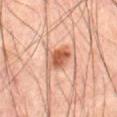Imaged during a routine full-body skin examination; the lesion was not biopsied and no histopathology is available.
Longest diameter approximately 3.5 mm.
Cropped from a whole-body photographic skin survey; the tile spans about 15 mm.
The lesion is located on the abdomen.
Imaged with cross-polarized lighting.
A male patient, in their mid-60s.